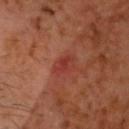site: the head or neck; patient: male, aged around 60; image: ~15 mm crop, total-body skin-cancer survey.